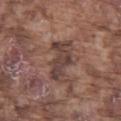Recorded during total-body skin imaging; not selected for excision or biopsy. The subject is a male aged around 75. A close-up tile cropped from a whole-body skin photograph, about 15 mm across. The lesion is located on the leg.